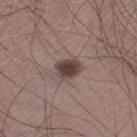<record>
  <biopsy_status>not biopsied; imaged during a skin examination</biopsy_status>
  <site>left thigh</site>
  <image>
    <source>total-body photography crop</source>
    <field_of_view_mm>15</field_of_view_mm>
  </image>
  <patient>
    <sex>male</sex>
    <age_approx>35</age_approx>
  </patient>
</record>